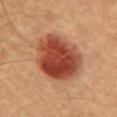biopsy status: imaged on a skin check; not biopsied | body site: the chest | image: total-body-photography crop, ~15 mm field of view | patient: male, approximately 65 years of age.